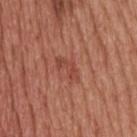Cropped from a total-body skin-imaging series; the visible field is about 15 mm. A male subject, in their 60s. On the upper back.